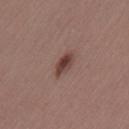Assessment:
Captured during whole-body skin photography for melanoma surveillance; the lesion was not biopsied.
Image and clinical context:
A 15 mm close-up extracted from a 3D total-body photography capture. This is a white-light tile. Longest diameter approximately 3 mm. A female patient in their mid-40s. The lesion-visualizer software estimated an area of roughly 4.5 mm², an outline eccentricity of about 0.8 (0 = round, 1 = elongated), and a symmetry-axis asymmetry near 0.2. It also reported a color-variation rating of about 3.5/10 and a peripheral color-asymmetry measure near 1. The software also gave a nevus-likeness score of about 95/100 and lesion-presence confidence of about 100/100. The lesion is on the left thigh.